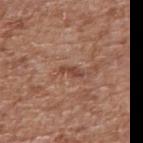Impression: The lesion was photographed on a routine skin check and not biopsied; there is no pathology result. Acquisition and patient details: A region of skin cropped from a whole-body photographic capture, roughly 15 mm wide. Approximately 3 mm at its widest. This is a white-light tile. On the right upper arm. A male patient aged approximately 70.